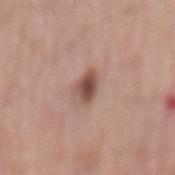workup — no biopsy performed (imaged during a skin exam)
subject — male, approximately 70 years of age
image — total-body-photography crop, ~15 mm field of view
site — the mid back